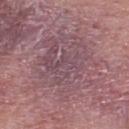workup: no biopsy performed (imaged during a skin exam) | patient: male, approximately 55 years of age | tile lighting: white-light | body site: the back | acquisition: total-body-photography crop, ~15 mm field of view | size: ~8.5 mm (longest diameter).